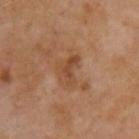Part of a total-body skin-imaging series; this lesion was reviewed on a skin check and was not flagged for biopsy. The lesion is located on the chest. A male patient, in their mid-50s. About 3.5 mm across. The tile uses cross-polarized illumination. A lesion tile, about 15 mm wide, cut from a 3D total-body photograph.